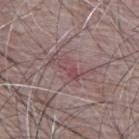Q: Is there a histopathology result?
A: catalogued during a skin exam; not biopsied
Q: Patient demographics?
A: male, aged 63–67
Q: What is the lesion's diameter?
A: ≈3 mm
Q: What is the anatomic site?
A: the upper back
Q: What lighting was used for the tile?
A: white-light illumination
Q: How was this image acquired?
A: ~15 mm tile from a whole-body skin photo
Q: Automated lesion metrics?
A: a border-irregularity index near 4/10, a color-variation rating of about 2/10, and peripheral color asymmetry of about 0.5